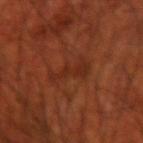Q: Was this lesion biopsied?
A: no biopsy performed (imaged during a skin exam)
Q: How was this image acquired?
A: ~15 mm crop, total-body skin-cancer survey
Q: What is the lesion's diameter?
A: about 4 mm
Q: How was the tile lit?
A: cross-polarized
Q: What is the anatomic site?
A: the right upper arm
Q: What did automated image analysis measure?
A: a footprint of about 7 mm², an eccentricity of roughly 0.85, and two-axis asymmetry of about 0.45; a nevus-likeness score of about 0/100 and a lesion-detection confidence of about 100/100
Q: What are the patient's age and sex?
A: male, about 70 years old